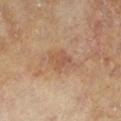biopsy status: no biopsy performed (imaged during a skin exam) | illumination: cross-polarized illumination | site: the left lower leg | lesion diameter: ~3 mm (longest diameter) | patient: male, about 65 years old | imaging modality: 15 mm crop, total-body photography.